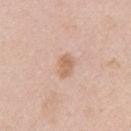The lesion was photographed on a routine skin check and not biopsied; there is no pathology result. A female patient aged around 40. A region of skin cropped from a whole-body photographic capture, roughly 15 mm wide. Located on the left upper arm. The lesion's longest dimension is about 3 mm.